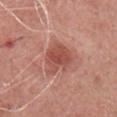{
  "biopsy_status": "not biopsied; imaged during a skin examination",
  "lighting": "white-light",
  "patient": {
    "sex": "male",
    "age_approx": 55
  },
  "site": "front of the torso",
  "automated_metrics": {
    "area_mm2_approx": 11.0,
    "eccentricity": 0.6,
    "shape_asymmetry": 0.3,
    "cielab_L": 51,
    "cielab_a": 28,
    "cielab_b": 28,
    "vs_skin_contrast_norm": 7.5,
    "border_irregularity_0_10": 3.5,
    "color_variation_0_10": 4.0,
    "peripheral_color_asymmetry": 1.0
  },
  "image": {
    "source": "total-body photography crop",
    "field_of_view_mm": 15
  }
}